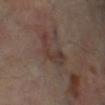Assessment: No biopsy was performed on this lesion — it was imaged during a full skin examination and was not determined to be concerning. Context: A male subject, approximately 70 years of age. A 15 mm close-up tile from a total-body photography series done for melanoma screening. On the right lower leg.